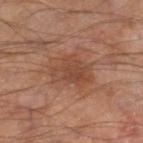The lesion was tiled from a total-body skin photograph and was not biopsied. About 5 mm across. The tile uses cross-polarized illumination. A male patient, in their mid- to late 60s. The lesion-visualizer software estimated a lesion color around L≈44 a*≈21 b*≈29 in CIELAB, roughly 7 lightness units darker than nearby skin, and a lesion-to-skin contrast of about 6 (normalized; higher = more distinct). Cropped from a whole-body photographic skin survey; the tile spans about 15 mm. The lesion is on the leg.